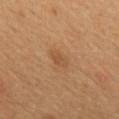{
  "biopsy_status": "not biopsied; imaged during a skin examination",
  "patient": {
    "sex": "male",
    "age_approx": 55
  },
  "site": "mid back",
  "lighting": "cross-polarized",
  "lesion_size": {
    "long_diameter_mm_approx": 2.5
  },
  "image": {
    "source": "total-body photography crop",
    "field_of_view_mm": 15
  }
}